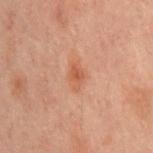Case summary:
* follow-up · catalogued during a skin exam; not biopsied
* image source · ~15 mm tile from a whole-body skin photo
* lesion diameter · ~3 mm (longest diameter)
* anatomic site · the chest
* subject · male, approximately 60 years of age
* tile lighting · cross-polarized